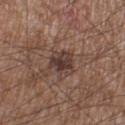Q: Was a biopsy performed?
A: catalogued during a skin exam; not biopsied
Q: What is the anatomic site?
A: the right lower leg
Q: Who is the patient?
A: male, approximately 55 years of age
Q: What kind of image is this?
A: ~15 mm tile from a whole-body skin photo